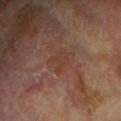{
  "biopsy_status": "not biopsied; imaged during a skin examination",
  "lesion_size": {
    "long_diameter_mm_approx": 2.5
  },
  "site": "right forearm",
  "image": {
    "source": "total-body photography crop",
    "field_of_view_mm": 15
  },
  "patient": {
    "sex": "female",
    "age_approx": 75
  },
  "automated_metrics": {
    "vs_skin_darker_L": 4.0,
    "vs_skin_contrast_norm": 5.0,
    "border_irregularity_0_10": 4.0,
    "color_variation_0_10": 1.5,
    "nevus_likeness_0_100": 0,
    "lesion_detection_confidence_0_100": 100
  },
  "lighting": "cross-polarized"
}